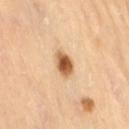Recorded during total-body skin imaging; not selected for excision or biopsy. A close-up tile cropped from a whole-body skin photograph, about 15 mm across. The lesion is located on the right thigh. Imaged with cross-polarized lighting. A female patient, aged approximately 70. The lesion's longest dimension is about 3 mm.